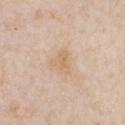Case summary:
- follow-up — total-body-photography surveillance lesion; no biopsy
- site — the chest
- image source — total-body-photography crop, ~15 mm field of view
- automated lesion analysis — a footprint of about 4.5 mm² and a symmetry-axis asymmetry near 0.45; an average lesion color of about L≈67 a*≈16 b*≈34 (CIELAB) and a normalized lesion–skin contrast near 5.5; an automated nevus-likeness rating near 0 out of 100 and a detector confidence of about 100 out of 100 that the crop contains a lesion
- subject — female, in their mid-40s
- lesion size — ~3 mm (longest diameter)